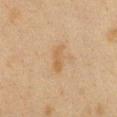Notes:
• tile lighting — cross-polarized
• location — the front of the torso
• patient — female, aged 38–42
• image source — 15 mm crop, total-body photography